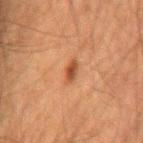biopsy_status: not biopsied; imaged during a skin examination
image:
  source: total-body photography crop
  field_of_view_mm: 15
lesion_size:
  long_diameter_mm_approx: 2.5
automated_metrics:
  area_mm2_approx: 3.0
  eccentricity: 0.85
  vs_skin_darker_L: 10.0
  vs_skin_contrast_norm: 8.0
patient:
  sex: male
  age_approx: 60
site: mid back